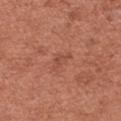Q: Is there a histopathology result?
A: total-body-photography surveillance lesion; no biopsy
Q: Lesion location?
A: the left forearm
Q: Illumination type?
A: white-light illumination
Q: What is the imaging modality?
A: 15 mm crop, total-body photography
Q: Lesion size?
A: ~3 mm (longest diameter)
Q: What are the patient's age and sex?
A: female, roughly 50 years of age